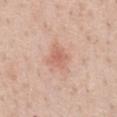  biopsy_status: not biopsied; imaged during a skin examination
  image:
    source: total-body photography crop
    field_of_view_mm: 15
  lesion_size:
    long_diameter_mm_approx: 2.5
  patient:
    sex: female
    age_approx: 45
  site: chest
  lighting: white-light